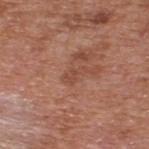notes: no biopsy performed (imaged during a skin exam); acquisition: ~15 mm tile from a whole-body skin photo; subject: male, roughly 65 years of age; lesion diameter: ≈2.5 mm; site: the upper back.